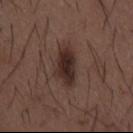Captured during whole-body skin photography for melanoma surveillance; the lesion was not biopsied. The lesion is located on the mid back. The patient is a male in their 50s. This image is a 15 mm lesion crop taken from a total-body photograph. Automated tile analysis of the lesion measured a border-irregularity rating of about 3/10, internal color variation of about 4.5 on a 0–10 scale, and peripheral color asymmetry of about 1.5. The analysis additionally found an automated nevus-likeness rating near 90 out of 100 and a detector confidence of about 100 out of 100 that the crop contains a lesion. Measured at roughly 5 mm in maximum diameter. The tile uses white-light illumination.